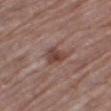{"biopsy_status": "not biopsied; imaged during a skin examination", "lighting": "white-light", "patient": {"sex": "male", "age_approx": 70}, "automated_metrics": {"cielab_L": 43, "cielab_a": 19, "cielab_b": 23, "vs_skin_darker_L": 10.0, "vs_skin_contrast_norm": 8.0, "border_irregularity_0_10": 3.0, "color_variation_0_10": 4.5, "peripheral_color_asymmetry": 1.5}, "site": "right thigh", "image": {"source": "total-body photography crop", "field_of_view_mm": 15}}